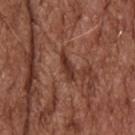No biopsy was performed on this lesion — it was imaged during a full skin examination and was not determined to be concerning. Captured under white-light illumination. Approximately 3.5 mm at its widest. A 15 mm close-up extracted from a 3D total-body photography capture. The lesion is on the head or neck. The patient is a male in their mid- to late 70s.